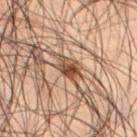The lesion was tiled from a total-body skin photograph and was not biopsied. The lesion is on the leg. A male subject, aged 48 to 52. A region of skin cropped from a whole-body photographic capture, roughly 15 mm wide.